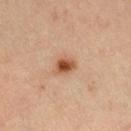Q: Is there a histopathology result?
A: total-body-photography surveillance lesion; no biopsy
Q: What is the lesion's diameter?
A: ~2.5 mm (longest diameter)
Q: Patient demographics?
A: female, roughly 50 years of age
Q: Where on the body is the lesion?
A: the right lower leg
Q: What lighting was used for the tile?
A: cross-polarized
Q: What is the imaging modality?
A: 15 mm crop, total-body photography